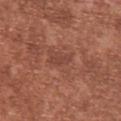image: total-body-photography crop, ~15 mm field of view; subject: male, roughly 45 years of age; location: the chest; lighting: white-light illumination.